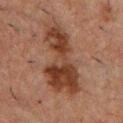The lesion was tiled from a total-body skin photograph and was not biopsied.
Automated tile analysis of the lesion measured an average lesion color of about L≈30 a*≈18 b*≈24 (CIELAB), about 10 CIELAB-L* units darker than the surrounding skin, and a lesion-to-skin contrast of about 9.5 (normalized; higher = more distinct). The analysis additionally found internal color variation of about 4.5 on a 0–10 scale. The software also gave a nevus-likeness score of about 0/100.
A lesion tile, about 15 mm wide, cut from a 3D total-body photograph.
The lesion's longest dimension is about 9.5 mm.
A male patient, roughly 50 years of age.
The lesion is on the chest.